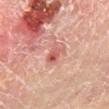Impression: Recorded during total-body skin imaging; not selected for excision or biopsy. Image and clinical context: The lesion is located on the right lower leg. A region of skin cropped from a whole-body photographic capture, roughly 15 mm wide. The patient is a male about 55 years old. Imaged with cross-polarized lighting.